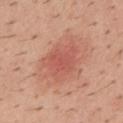Impression:
Captured during whole-body skin photography for melanoma surveillance; the lesion was not biopsied.
Acquisition and patient details:
Imaged with white-light lighting. The total-body-photography lesion software estimated a footprint of about 4.5 mm², an outline eccentricity of about 0.7 (0 = round, 1 = elongated), and a shape-asymmetry score of about 0.35 (0 = symmetric). The software also gave an average lesion color of about L≈54 a*≈32 b*≈29 (CIELAB) and a normalized lesion–skin contrast near 4.5. A male subject approximately 40 years of age. Measured at roughly 2.5 mm in maximum diameter. The lesion is on the mid back. A roughly 15 mm field-of-view crop from a total-body skin photograph.